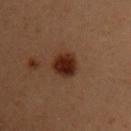Assessment:
Captured during whole-body skin photography for melanoma surveillance; the lesion was not biopsied.
Context:
The patient is a female aged approximately 40. On the chest. A 15 mm close-up tile from a total-body photography series done for melanoma screening.